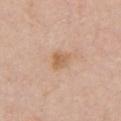Notes:
• workup: total-body-photography surveillance lesion; no biopsy
• automated lesion analysis: about 8 CIELAB-L* units darker than the surrounding skin; a border-irregularity rating of about 3/10, a color-variation rating of about 3/10, and peripheral color asymmetry of about 1; a nevus-likeness score of about 10/100 and a detector confidence of about 100 out of 100 that the crop contains a lesion
• anatomic site: the chest
• lesion size: about 2.5 mm
• lighting: white-light
• image: total-body-photography crop, ~15 mm field of view
• subject: female, aged 53–57